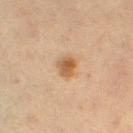notes = no biopsy performed (imaged during a skin exam)
anatomic site = the leg
patient = female, roughly 40 years of age
tile lighting = cross-polarized
diameter = ~2.5 mm (longest diameter)
image = ~15 mm crop, total-body skin-cancer survey
TBP lesion metrics = a lesion area of about 5 mm², an eccentricity of roughly 0.55, and two-axis asymmetry of about 0.15; an average lesion color of about L≈47 a*≈16 b*≈32 (CIELAB) and about 9 CIELAB-L* units darker than the surrounding skin; a classifier nevus-likeness of about 95/100 and lesion-presence confidence of about 100/100